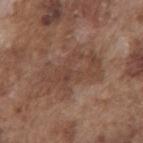- patient — male, about 75 years old
- lesion diameter — ≈7 mm
- automated lesion analysis — an average lesion color of about L≈44 a*≈18 b*≈26 (CIELAB), roughly 6 lightness units darker than nearby skin, and a normalized border contrast of about 5; a border-irregularity index near 6/10, a color-variation rating of about 4/10, and peripheral color asymmetry of about 1.5
- acquisition — ~15 mm crop, total-body skin-cancer survey
- anatomic site — the front of the torso